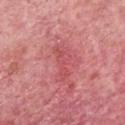{"biopsy_status": "not biopsied; imaged during a skin examination", "patient": {"sex": "female", "age_approx": 60}, "image": {"source": "total-body photography crop", "field_of_view_mm": 15}, "site": "head or neck"}